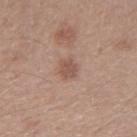Case summary:
- biopsy status: no biopsy performed (imaged during a skin exam)
- size: ~2.5 mm (longest diameter)
- subject: male, aged around 30
- automated metrics: radial color variation of about 0.5
- acquisition: ~15 mm tile from a whole-body skin photo